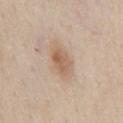No biopsy was performed on this lesion — it was imaged during a full skin examination and was not determined to be concerning.
Located on the chest.
A 15 mm close-up tile from a total-body photography series done for melanoma screening.
A male patient approximately 60 years of age.